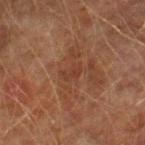biopsy status: total-body-photography surveillance lesion; no biopsy | image source: 15 mm crop, total-body photography | subject: male, aged approximately 75 | illumination: cross-polarized | size: ≈2.5 mm | location: the right lower leg.